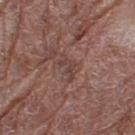Impression:
The lesion was tiled from a total-body skin photograph and was not biopsied.
Acquisition and patient details:
Imaged with white-light lighting. Located on the left thigh. The lesion-visualizer software estimated a footprint of about 4 mm², an eccentricity of roughly 0.9, and a shape-asymmetry score of about 0.55 (0 = symmetric). It also reported a lesion–skin lightness drop of about 7 and a normalized lesion–skin contrast near 5.5. And it measured a border-irregularity rating of about 7.5/10, a color-variation rating of about 0/10, and a peripheral color-asymmetry measure near 0. Longest diameter approximately 3.5 mm. A close-up tile cropped from a whole-body skin photograph, about 15 mm across. The patient is a female roughly 80 years of age.